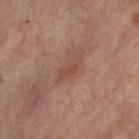Notes:
– follow-up — total-body-photography surveillance lesion; no biopsy
– imaging modality — total-body-photography crop, ~15 mm field of view
– location — the right thigh
– illumination — cross-polarized
– subject — female, aged approximately 55